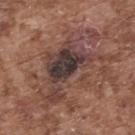Q: Was a biopsy performed?
A: no biopsy performed (imaged during a skin exam)
Q: What is the anatomic site?
A: the upper back
Q: What is the imaging modality?
A: total-body-photography crop, ~15 mm field of view
Q: Lesion size?
A: ~10 mm (longest diameter)
Q: Patient demographics?
A: male, aged 73 to 77
Q: What lighting was used for the tile?
A: white-light illumination
Q: What did automated image analysis measure?
A: an average lesion color of about L≈39 a*≈17 b*≈20 (CIELAB), a lesion–skin lightness drop of about 11, and a lesion-to-skin contrast of about 10 (normalized; higher = more distinct); border irregularity of about 9.5 on a 0–10 scale, internal color variation of about 7.5 on a 0–10 scale, and a peripheral color-asymmetry measure near 1.5; a lesion-detection confidence of about 60/100